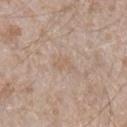Case summary:
- biopsy status: imaged on a skin check; not biopsied
- image source: 15 mm crop, total-body photography
- diameter: ≈2.5 mm
- illumination: white-light
- subject: male, in their mid-40s
- body site: the leg